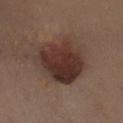This lesion was catalogued during total-body skin photography and was not selected for biopsy. The patient is a female approximately 40 years of age. This image is a 15 mm lesion crop taken from a total-body photograph. The total-body-photography lesion software estimated an area of roughly 25 mm², an eccentricity of roughly 0.6, and a symmetry-axis asymmetry near 0.2. The software also gave a lesion color around L≈33 a*≈18 b*≈22 in CIELAB, about 12 CIELAB-L* units darker than the surrounding skin, and a normalized border contrast of about 10.5. The lesion's longest dimension is about 6 mm. The lesion is located on the left leg.